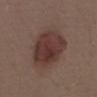Clinical impression: Part of a total-body skin-imaging series; this lesion was reviewed on a skin check and was not flagged for biopsy. Context: About 5.5 mm across. The patient is a female in their mid- to late 30s. Automated image analysis of the tile measured an area of roughly 23 mm² and a shape eccentricity near 0.25. The analysis additionally found a border-irregularity index near 1/10, internal color variation of about 4 on a 0–10 scale, and a peripheral color-asymmetry measure near 1.5. On the chest. A 15 mm close-up tile from a total-body photography series done for melanoma screening.